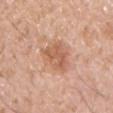Case summary:
– workup · imaged on a skin check; not biopsied
– location · the chest
– imaging modality · ~15 mm crop, total-body skin-cancer survey
– patient · male, in their 40s